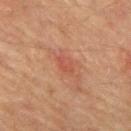Impression:
Recorded during total-body skin imaging; not selected for excision or biopsy.
Image and clinical context:
A male patient aged approximately 75. Located on the mid back. The lesion-visualizer software estimated an outline eccentricity of about 0.8 (0 = round, 1 = elongated) and a symmetry-axis asymmetry near 0.25. And it measured internal color variation of about 1.5 on a 0–10 scale and a peripheral color-asymmetry measure near 0.5. And it measured an automated nevus-likeness rating near 10 out of 100 and a lesion-detection confidence of about 100/100. The tile uses cross-polarized illumination. Cropped from a whole-body photographic skin survey; the tile spans about 15 mm.